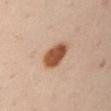The lesion was photographed on a routine skin check and not biopsied; there is no pathology result.
Cropped from a total-body skin-imaging series; the visible field is about 15 mm.
Captured under cross-polarized illumination.
The total-body-photography lesion software estimated border irregularity of about 1.5 on a 0–10 scale, a color-variation rating of about 3/10, and a peripheral color-asymmetry measure near 1.
The subject is a male aged around 50.
About 4 mm across.
The lesion is on the left upper arm.